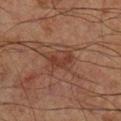Recorded during total-body skin imaging; not selected for excision or biopsy. This image is a 15 mm lesion crop taken from a total-body photograph. The lesion is located on the leg. Imaged with cross-polarized lighting. A male patient, aged approximately 70. Approximately 3 mm at its widest.